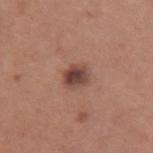The lesion was photographed on a routine skin check and not biopsied; there is no pathology result.
A lesion tile, about 15 mm wide, cut from a 3D total-body photograph.
A female subject roughly 30 years of age.
About 2.5 mm across.
The lesion is located on the left upper arm.
Imaged with white-light lighting.